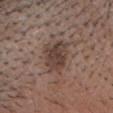Imaged during a routine full-body skin examination; the lesion was not biopsied and no histopathology is available. A male patient, aged around 65. Approximately 4.5 mm at its widest. An algorithmic analysis of the crop reported a lesion–skin lightness drop of about 10 and a normalized lesion–skin contrast near 8.5. And it measured peripheral color asymmetry of about 1. And it measured a nevus-likeness score of about 55/100. A roughly 15 mm field-of-view crop from a total-body skin photograph. From the head or neck. This is a white-light tile.